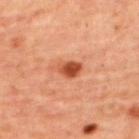Captured during whole-body skin photography for melanoma surveillance; the lesion was not biopsied.
The lesion is located on the upper back.
Imaged with cross-polarized lighting.
A close-up tile cropped from a whole-body skin photograph, about 15 mm across.
Automated tile analysis of the lesion measured an average lesion color of about L≈51 a*≈31 b*≈38 (CIELAB), a lesion–skin lightness drop of about 14, and a normalized lesion–skin contrast near 10. The analysis additionally found a nevus-likeness score of about 95/100 and a lesion-detection confidence of about 100/100.
A female patient in their mid- to late 40s.
Longest diameter approximately 3 mm.